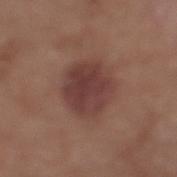Case summary:
– notes — imaged on a skin check; not biopsied